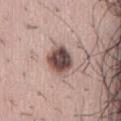Recorded during total-body skin imaging; not selected for excision or biopsy. The subject is a female about 50 years old. Captured under white-light illumination. Measured at roughly 4 mm in maximum diameter. From the abdomen. A 15 mm close-up tile from a total-body photography series done for melanoma screening.